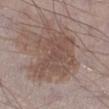Notes:
* workup: total-body-photography surveillance lesion; no biopsy
* diameter: ~7 mm (longest diameter)
* automated metrics: a footprint of about 30 mm² and an outline eccentricity of about 0.25 (0 = round, 1 = elongated); a lesion color around L≈49 a*≈16 b*≈23 in CIELAB, roughly 8 lightness units darker than nearby skin, and a normalized lesion–skin contrast near 6; an automated nevus-likeness rating near 35 out of 100 and a lesion-detection confidence of about 95/100
* lighting: white-light illumination
* subject: male, approximately 70 years of age
* anatomic site: the right lower leg
* acquisition: total-body-photography crop, ~15 mm field of view